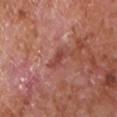Part of a total-body skin-imaging series; this lesion was reviewed on a skin check and was not flagged for biopsy. From the chest. A male subject, aged 63 to 67. A 15 mm crop from a total-body photograph taken for skin-cancer surveillance. Automated tile analysis of the lesion measured a lesion area of about 3.5 mm² and an eccentricity of roughly 0.9. And it measured a mean CIELAB color near L≈46 a*≈28 b*≈27, roughly 9 lightness units darker than nearby skin, and a normalized lesion–skin contrast near 7. The recorded lesion diameter is about 3 mm. Imaged with white-light lighting.